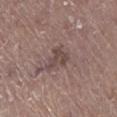Case summary:
• biopsy status · catalogued during a skin exam; not biopsied
• diameter · ~3.5 mm (longest diameter)
• image · 15 mm crop, total-body photography
• illumination · white-light illumination
• subject · male, aged 63–67
• body site · the left lower leg